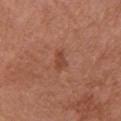  biopsy_status: not biopsied; imaged during a skin examination
  patient:
    sex: female
    age_approx: 65
  lesion_size:
    long_diameter_mm_approx: 2.5
  automated_metrics:
    border_irregularity_0_10: 4.0
    color_variation_0_10: 0.5
    peripheral_color_asymmetry: 0.0
    nevus_likeness_0_100: 0
    lesion_detection_confidence_0_100: 100
  lighting: white-light
  site: left upper arm
  image:
    source: total-body photography crop
    field_of_view_mm: 15